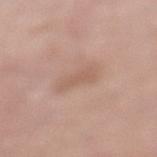Part of a total-body skin-imaging series; this lesion was reviewed on a skin check and was not flagged for biopsy.